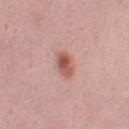Q: Was this lesion biopsied?
A: no biopsy performed (imaged during a skin exam)
Q: Who is the patient?
A: female, about 15 years old
Q: What is the imaging modality?
A: ~15 mm tile from a whole-body skin photo
Q: Where on the body is the lesion?
A: the abdomen